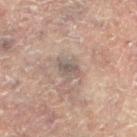Captured during whole-body skin photography for melanoma surveillance; the lesion was not biopsied. Approximately 2.5 mm at its widest. The lesion is on the right leg. The lesion-visualizer software estimated an area of roughly 3.5 mm², an outline eccentricity of about 0.8 (0 = round, 1 = elongated), and a shape-asymmetry score of about 0.25 (0 = symmetric). A female subject in their 80s. Imaged with cross-polarized lighting. A region of skin cropped from a whole-body photographic capture, roughly 15 mm wide.